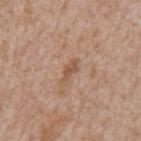Captured during whole-body skin photography for melanoma surveillance; the lesion was not biopsied.
Automated tile analysis of the lesion measured a footprint of about 3 mm² and an eccentricity of roughly 0.9. The software also gave a border-irregularity rating of about 4/10, a within-lesion color-variation index near 0/10, and peripheral color asymmetry of about 0. And it measured a classifier nevus-likeness of about 0/100 and lesion-presence confidence of about 100/100.
On the mid back.
Cropped from a total-body skin-imaging series; the visible field is about 15 mm.
This is a white-light tile.
The recorded lesion diameter is about 3 mm.
A male patient aged around 65.